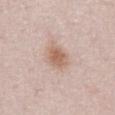Captured during whole-body skin photography for melanoma surveillance; the lesion was not biopsied. Cropped from a total-body skin-imaging series; the visible field is about 15 mm. The lesion's longest dimension is about 3 mm. The subject is a female about 50 years old. The lesion-visualizer software estimated an average lesion color of about L≈61 a*≈19 b*≈28 (CIELAB), a lesion–skin lightness drop of about 10, and a normalized lesion–skin contrast near 7.5. The software also gave a classifier nevus-likeness of about 90/100 and a lesion-detection confidence of about 100/100. Located on the abdomen.